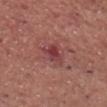<lesion>
<biopsy_status>not biopsied; imaged during a skin examination</biopsy_status>
<automated_metrics>
  <area_mm2_approx>4.5</area_mm2_approx>
  <shape_asymmetry>0.35</shape_asymmetry>
  <cielab_L>41</cielab_L>
  <cielab_a>30</cielab_a>
  <cielab_b>21</cielab_b>
  <vs_skin_darker_L>9.0</vs_skin_darker_L>
  <vs_skin_contrast_norm>7.5</vs_skin_contrast_norm>
</automated_metrics>
<lighting>white-light</lighting>
<patient>
  <sex>male</sex>
  <age_approx>70</age_approx>
</patient>
<lesion_size>
  <long_diameter_mm_approx>3.0</long_diameter_mm_approx>
</lesion_size>
<image>
  <source>total-body photography crop</source>
  <field_of_view_mm>15</field_of_view_mm>
</image>
<site>chest</site>
</lesion>